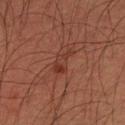Findings:
• notes · total-body-photography surveillance lesion; no biopsy
• image-analysis metrics · an area of roughly 4 mm², an outline eccentricity of about 0.85 (0 = round, 1 = elongated), and a shape-asymmetry score of about 0.45 (0 = symmetric); a lesion color around L≈33 a*≈23 b*≈26 in CIELAB and about 6 CIELAB-L* units darker than the surrounding skin; a nevus-likeness score of about 5/100 and a detector confidence of about 100 out of 100 that the crop contains a lesion
• lighting · cross-polarized
• subject · male, approximately 35 years of age
• image · total-body-photography crop, ~15 mm field of view
• body site · the left forearm